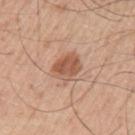The lesion is on the left upper arm. A roughly 15 mm field-of-view crop from a total-body skin photograph. The subject is a male aged 53–57. The total-body-photography lesion software estimated an area of roughly 8 mm², an outline eccentricity of about 0.65 (0 = round, 1 = elongated), and two-axis asymmetry of about 0.15.